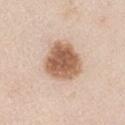Findings:
- workup: total-body-photography surveillance lesion; no biopsy
- illumination: white-light
- automated metrics: an automated nevus-likeness rating near 95 out of 100 and a lesion-detection confidence of about 100/100
- acquisition: 15 mm crop, total-body photography
- anatomic site: the right upper arm
- size: ~5 mm (longest diameter)
- subject: male, aged 43–47